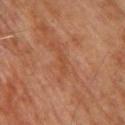<lesion>
  <biopsy_status>not biopsied; imaged during a skin examination</biopsy_status>
  <lighting>cross-polarized</lighting>
  <site>back</site>
  <image>
    <source>total-body photography crop</source>
    <field_of_view_mm>15</field_of_view_mm>
  </image>
  <patient>
    <sex>male</sex>
    <age_approx>75</age_approx>
  </patient>
  <lesion_size>
    <long_diameter_mm_approx>3.0</long_diameter_mm_approx>
  </lesion_size>
</lesion>